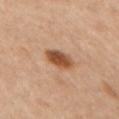• follow-up: total-body-photography surveillance lesion; no biopsy
• imaging modality: 15 mm crop, total-body photography
• lesion size: ~3.5 mm (longest diameter)
• subject: female, about 60 years old
• anatomic site: the upper back
• TBP lesion metrics: a lesion area of about 7 mm², an outline eccentricity of about 0.7 (0 = round, 1 = elongated), and a symmetry-axis asymmetry near 0.15
• tile lighting: cross-polarized illumination